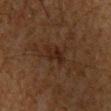<tbp_lesion>
  <biopsy_status>not biopsied; imaged during a skin examination</biopsy_status>
  <patient>
    <sex>male</sex>
    <age_approx>65</age_approx>
  </patient>
  <lesion_size>
    <long_diameter_mm_approx>3.0</long_diameter_mm_approx>
  </lesion_size>
  <image>
    <source>total-body photography crop</source>
    <field_of_view_mm>15</field_of_view_mm>
  </image>
  <site>chest</site>
</tbp_lesion>